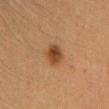No biopsy was performed on this lesion — it was imaged during a full skin examination and was not determined to be concerning. An algorithmic analysis of the crop reported a lesion area of about 5.5 mm², a shape eccentricity near 0.55, and a shape-asymmetry score of about 0.1 (0 = symmetric). The software also gave a lesion color around L≈37 a*≈19 b*≈31 in CIELAB, roughly 10 lightness units darker than nearby skin, and a lesion-to-skin contrast of about 9 (normalized; higher = more distinct). The software also gave a border-irregularity index near 1/10, a within-lesion color-variation index near 3.5/10, and a peripheral color-asymmetry measure near 1.5. The analysis additionally found a classifier nevus-likeness of about 100/100 and a detector confidence of about 100 out of 100 that the crop contains a lesion. The lesion is on the head or neck. Cropped from a total-body skin-imaging series; the visible field is about 15 mm. Longest diameter approximately 2.5 mm. A female patient aged approximately 40. The tile uses cross-polarized illumination.